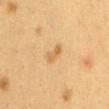Captured under cross-polarized illumination. A female patient, aged approximately 40. A 15 mm crop from a total-body photograph taken for skin-cancer surveillance. The lesion's longest dimension is about 2.5 mm. From the mid back.